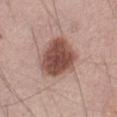No biopsy was performed on this lesion — it was imaged during a full skin examination and was not determined to be concerning. A roughly 15 mm field-of-view crop from a total-body skin photograph. A male subject, approximately 75 years of age. Captured under white-light illumination. The lesion is on the abdomen. About 5.5 mm across.